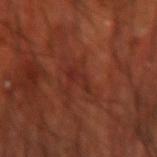Imaged during a routine full-body skin examination; the lesion was not biopsied and no histopathology is available.
A male patient aged 68–72.
About 3 mm across.
Cropped from a total-body skin-imaging series; the visible field is about 15 mm.
From the right forearm.
The lesion-visualizer software estimated a footprint of about 3 mm², an eccentricity of roughly 0.9, and two-axis asymmetry of about 0.6. And it measured an average lesion color of about L≈27 a*≈26 b*≈27 (CIELAB), about 5 CIELAB-L* units darker than the surrounding skin, and a lesion-to-skin contrast of about 5.5 (normalized; higher = more distinct). It also reported a classifier nevus-likeness of about 0/100 and a detector confidence of about 55 out of 100 that the crop contains a lesion.